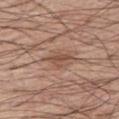Clinical impression:
No biopsy was performed on this lesion — it was imaged during a full skin examination and was not determined to be concerning.
Acquisition and patient details:
From the right thigh. A 15 mm close-up tile from a total-body photography series done for melanoma screening. This is a white-light tile. A male patient, aged approximately 60. Approximately 3 mm at its widest. The lesion-visualizer software estimated a lesion area of about 4 mm², an eccentricity of roughly 0.75, and two-axis asymmetry of about 0.4. And it measured a border-irregularity rating of about 4/10 and peripheral color asymmetry of about 1.5.